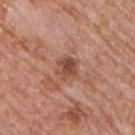The lesion was photographed on a routine skin check and not biopsied; there is no pathology result. Automated image analysis of the tile measured an outline eccentricity of about 0.15 (0 = round, 1 = elongated) and a shape-asymmetry score of about 0.25 (0 = symmetric). It also reported a mean CIELAB color near L≈48 a*≈24 b*≈29 and a lesion–skin lightness drop of about 11. And it measured border irregularity of about 3 on a 0–10 scale, a within-lesion color-variation index near 4/10, and peripheral color asymmetry of about 1.5. The software also gave a classifier nevus-likeness of about 15/100. The patient is a male about 75 years old. A 15 mm crop from a total-body photograph taken for skin-cancer surveillance. Captured under white-light illumination. On the chest.